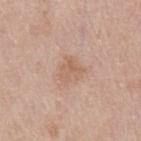notes — imaged on a skin check; not biopsied
automated metrics — an outline eccentricity of about 0.45 (0 = round, 1 = elongated) and a shape-asymmetry score of about 0.3 (0 = symmetric)
diameter — ~3 mm (longest diameter)
acquisition — ~15 mm tile from a whole-body skin photo
patient — male, aged 58–62
anatomic site — the abdomen